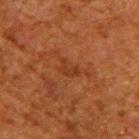Image and clinical context:
A male patient, in their 60s. This is a cross-polarized tile. The recorded lesion diameter is about 2.5 mm. Located on the upper back. Automated tile analysis of the lesion measured roughly 5 lightness units darker than nearby skin and a normalized border contrast of about 5.5. A 15 mm crop from a total-body photograph taken for skin-cancer surveillance.